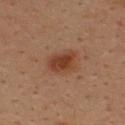Q: Was a biopsy performed?
A: imaged on a skin check; not biopsied
Q: How was this image acquired?
A: total-body-photography crop, ~15 mm field of view
Q: What is the lesion's diameter?
A: ~3.5 mm (longest diameter)
Q: Illumination type?
A: cross-polarized illumination
Q: What is the anatomic site?
A: the upper back
Q: Patient demographics?
A: male, in their mid-30s